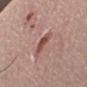Clinical impression:
No biopsy was performed on this lesion — it was imaged during a full skin examination and was not determined to be concerning.
Clinical summary:
Imaged with white-light lighting. Located on the arm. A close-up tile cropped from a whole-body skin photograph, about 15 mm across. A male patient approximately 65 years of age. About 4 mm across.